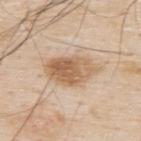| field | value |
|---|---|
| notes | total-body-photography surveillance lesion; no biopsy |
| anatomic site | the upper back |
| automated lesion analysis | a mean CIELAB color near L≈62 a*≈17 b*≈34 and about 12 CIELAB-L* units darker than the surrounding skin; border irregularity of about 2 on a 0–10 scale, a color-variation rating of about 6.5/10, and peripheral color asymmetry of about 2.5 |
| acquisition | total-body-photography crop, ~15 mm field of view |
| subject | male, about 80 years old |